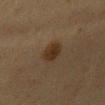workup: no biopsy performed (imaged during a skin exam)
diameter: ≈2.5 mm
site: the chest
lighting: cross-polarized illumination
subject: female, aged 48 to 52
imaging modality: total-body-photography crop, ~15 mm field of view